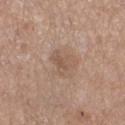Located on the left lower leg.
A female subject aged 53–57.
Automated image analysis of the tile measured a nevus-likeness score of about 0/100 and a detector confidence of about 100 out of 100 that the crop contains a lesion.
A close-up tile cropped from a whole-body skin photograph, about 15 mm across.